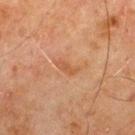| field | value |
|---|---|
| workup | catalogued during a skin exam; not biopsied |
| lighting | cross-polarized illumination |
| acquisition | ~15 mm crop, total-body skin-cancer survey |
| lesion diameter | ≈3 mm |
| site | the upper back |
| patient | male, approximately 70 years of age |
| automated lesion analysis | an eccentricity of roughly 0.9; roughly 6 lightness units darker than nearby skin and a normalized lesion–skin contrast near 5.5; a border-irregularity index near 5/10, a within-lesion color-variation index near 0/10, and peripheral color asymmetry of about 0 |